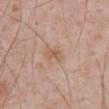Impression: This lesion was catalogued during total-body skin photography and was not selected for biopsy. Image and clinical context: On the abdomen. A close-up tile cropped from a whole-body skin photograph, about 15 mm across. The lesion's longest dimension is about 2.5 mm. A male subject, aged 68–72. Automated tile analysis of the lesion measured a mean CIELAB color near L≈58 a*≈19 b*≈30 and a normalized border contrast of about 6. The analysis additionally found a border-irregularity rating of about 3/10 and a color-variation rating of about 1/10. The software also gave a nevus-likeness score of about 0/100 and a detector confidence of about 100 out of 100 that the crop contains a lesion. This is a white-light tile.